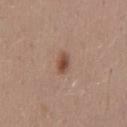biopsy_status: not biopsied; imaged during a skin examination
image:
  source: total-body photography crop
  field_of_view_mm: 15
lighting: white-light
lesion_size:
  long_diameter_mm_approx: 2.5
patient:
  sex: female
  age_approx: 40
site: mid back